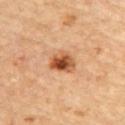<tbp_lesion>
<biopsy_status>not biopsied; imaged during a skin examination</biopsy_status>
<site>upper back</site>
<automated_metrics>
  <border_irregularity_0_10>2.0</border_irregularity_0_10>
  <color_variation_0_10>8.5</color_variation_0_10>
  <peripheral_color_asymmetry>2.5</peripheral_color_asymmetry>
</automated_metrics>
<lighting>cross-polarized</lighting>
<image>
  <source>total-body photography crop</source>
  <field_of_view_mm>15</field_of_view_mm>
</image>
<lesion_size>
  <long_diameter_mm_approx>3.0</long_diameter_mm_approx>
</lesion_size>
<patient>
  <sex>male</sex>
  <age_approx>85+</age_approx>
</patient>
</tbp_lesion>